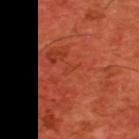The lesion was photographed on a routine skin check and not biopsied; there is no pathology result. Captured under cross-polarized illumination. This image is a 15 mm lesion crop taken from a total-body photograph. The subject is a male aged approximately 60. From the upper back. The total-body-photography lesion software estimated border irregularity of about 4.5 on a 0–10 scale, a within-lesion color-variation index near 0/10, and peripheral color asymmetry of about 0. The analysis additionally found a nevus-likeness score of about 0/100. The recorded lesion diameter is about 1.5 mm.